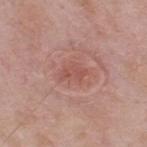Q: Is there a histopathology result?
A: imaged on a skin check; not biopsied
Q: What is the lesion's diameter?
A: ≈3 mm
Q: Where on the body is the lesion?
A: the back
Q: What kind of image is this?
A: ~15 mm crop, total-body skin-cancer survey
Q: Patient demographics?
A: male, aged approximately 55